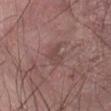biopsy status = no biopsy performed (imaged during a skin exam) | size = ≈3 mm | illumination = white-light | patient = male, in their 60s | site = the abdomen | imaging modality = 15 mm crop, total-body photography.